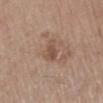– notes: no biopsy performed (imaged during a skin exam)
– patient: female, aged approximately 55
– lighting: white-light illumination
– diameter: about 2.5 mm
– image: ~15 mm crop, total-body skin-cancer survey
– site: the left lower leg